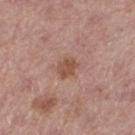{"biopsy_status": "not biopsied; imaged during a skin examination", "image": {"source": "total-body photography crop", "field_of_view_mm": 15}, "lesion_size": {"long_diameter_mm_approx": 2.5}, "lighting": "white-light", "site": "right thigh", "patient": {"sex": "male", "age_approx": 55}}